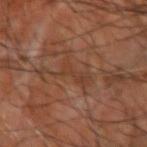Captured during whole-body skin photography for melanoma surveillance; the lesion was not biopsied. A subject aged around 65. This image is a 15 mm lesion crop taken from a total-body photograph. Located on the right upper arm.